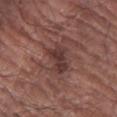{"biopsy_status": "not biopsied; imaged during a skin examination", "lighting": "white-light", "image": {"source": "total-body photography crop", "field_of_view_mm": 15}, "patient": {"sex": "male", "age_approx": 70}, "lesion_size": {"long_diameter_mm_approx": 3.5}, "site": "right forearm"}